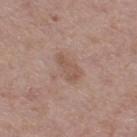| feature | finding |
|---|---|
| workup | no biopsy performed (imaged during a skin exam) |
| lesion size | about 3.5 mm |
| subject | female, in their 50s |
| anatomic site | the right thigh |
| illumination | white-light illumination |
| image source | 15 mm crop, total-body photography |